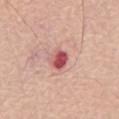The lesion was tiled from a total-body skin photograph and was not biopsied. About 2.5 mm across. A lesion tile, about 15 mm wide, cut from a 3D total-body photograph. The lesion is on the mid back. A male patient, approximately 70 years of age. This is a white-light tile.